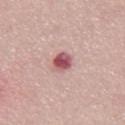<tbp_lesion>
<biopsy_status>not biopsied; imaged during a skin examination</biopsy_status>
<image>
  <source>total-body photography crop</source>
  <field_of_view_mm>15</field_of_view_mm>
</image>
<site>abdomen</site>
<lighting>white-light</lighting>
<lesion_size>
  <long_diameter_mm_approx>2.5</long_diameter_mm_approx>
</lesion_size>
<patient>
  <sex>male</sex>
  <age_approx>50</age_approx>
</patient>
</tbp_lesion>